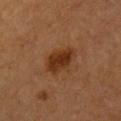This lesion was catalogued during total-body skin photography and was not selected for biopsy. Located on the chest. The subject is a female aged 38 to 42. A lesion tile, about 15 mm wide, cut from a 3D total-body photograph.